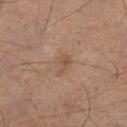{"biopsy_status": "not biopsied; imaged during a skin examination", "patient": {"sex": "male", "age_approx": 45}, "lesion_size": {"long_diameter_mm_approx": 2.5}, "site": "left lower leg", "lighting": "cross-polarized", "image": {"source": "total-body photography crop", "field_of_view_mm": 15}}